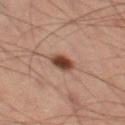This lesion was catalogued during total-body skin photography and was not selected for biopsy.
The recorded lesion diameter is about 3 mm.
A region of skin cropped from a whole-body photographic capture, roughly 15 mm wide.
The lesion-visualizer software estimated a border-irregularity rating of about 1.5/10, a within-lesion color-variation index near 3/10, and radial color variation of about 1.
The lesion is on the left thigh.
The patient is a male about 60 years old.